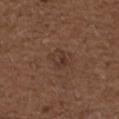The lesion was tiled from a total-body skin photograph and was not biopsied. The lesion-visualizer software estimated a mean CIELAB color near L≈35 a*≈18 b*≈24 and a lesion–skin lightness drop of about 6. From the left upper arm. A close-up tile cropped from a whole-body skin photograph, about 15 mm across. A male patient aged around 50. Approximately 2.5 mm at its widest. This is a white-light tile.